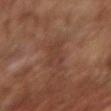<record>
  <biopsy_status>not biopsied; imaged during a skin examination</biopsy_status>
  <lesion_size>
    <long_diameter_mm_approx>5.5</long_diameter_mm_approx>
  </lesion_size>
  <site>right forearm</site>
  <image>
    <source>total-body photography crop</source>
    <field_of_view_mm>15</field_of_view_mm>
  </image>
  <automated_metrics>
    <border_irregularity_0_10>5.0</border_irregularity_0_10>
  </automated_metrics>
  <lighting>cross-polarized</lighting>
  <patient>
    <sex>female</sex>
    <age_approx>65</age_approx>
  </patient>
</record>